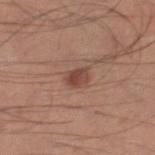Part of a total-body skin-imaging series; this lesion was reviewed on a skin check and was not flagged for biopsy. A male patient, aged 33–37. Captured under white-light illumination. Automated image analysis of the tile measured a footprint of about 4.5 mm², an eccentricity of roughly 0.7, and a shape-asymmetry score of about 0.25 (0 = symmetric). The software also gave border irregularity of about 2 on a 0–10 scale and radial color variation of about 1.5. A close-up tile cropped from a whole-body skin photograph, about 15 mm across. On the right lower leg. Longest diameter approximately 3 mm.